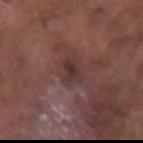This lesion was catalogued during total-body skin photography and was not selected for biopsy. The lesion is on the right forearm. Imaged with white-light lighting. Cropped from a total-body skin-imaging series; the visible field is about 15 mm. A male subject, about 75 years old. The lesion's longest dimension is about 3 mm.